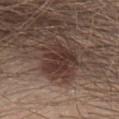Assessment: Imaged during a routine full-body skin examination; the lesion was not biopsied and no histopathology is available. Acquisition and patient details: The patient is a male aged around 40. This is a white-light tile. A 15 mm close-up tile from a total-body photography series done for melanoma screening. The lesion's longest dimension is about 4.5 mm. The lesion is on the chest.